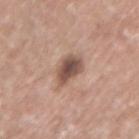notes: total-body-photography surveillance lesion; no biopsy
imaging modality: ~15 mm tile from a whole-body skin photo
site: the right upper arm
automated metrics: an outline eccentricity of about 0.45 (0 = round, 1 = elongated); a mean CIELAB color near L≈51 a*≈18 b*≈25 and a lesion-to-skin contrast of about 10 (normalized; higher = more distinct)
patient: male, aged around 70
lighting: white-light